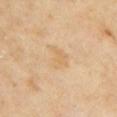Assessment:
Part of a total-body skin-imaging series; this lesion was reviewed on a skin check and was not flagged for biopsy.
Acquisition and patient details:
This is a cross-polarized tile. From the left upper arm. The subject is a female about 60 years old. Cropped from a whole-body photographic skin survey; the tile spans about 15 mm. The total-body-photography lesion software estimated a lesion area of about 6 mm², a shape eccentricity near 0.7, and two-axis asymmetry of about 0.4. And it measured a border-irregularity rating of about 4/10. The software also gave a detector confidence of about 100 out of 100 that the crop contains a lesion. Longest diameter approximately 3.5 mm.